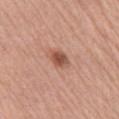notes = no biopsy performed (imaged during a skin exam) | location = the right upper arm | tile lighting = white-light | automated lesion analysis = a lesion area of about 4 mm², an eccentricity of roughly 0.65, and a symmetry-axis asymmetry near 0.25; border irregularity of about 2 on a 0–10 scale, a within-lesion color-variation index near 3.5/10, and a peripheral color-asymmetry measure near 1; a nevus-likeness score of about 90/100 | subject = female, aged 58 to 62 | lesion diameter = ≈2.5 mm | image = total-body-photography crop, ~15 mm field of view.